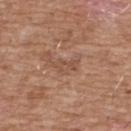follow-up: imaged on a skin check; not biopsied
anatomic site: the front of the torso
illumination: white-light illumination
image source: ~15 mm crop, total-body skin-cancer survey
size: about 3 mm
subject: male, about 60 years old